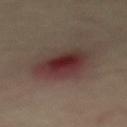The lesion was photographed on a routine skin check and not biopsied; there is no pathology result.
About 6 mm across.
The lesion is on the mid back.
Captured under cross-polarized illumination.
An algorithmic analysis of the crop reported a lesion area of about 19 mm², an eccentricity of roughly 0.75, and a symmetry-axis asymmetry near 0.2. The analysis additionally found an average lesion color of about L≈33 a*≈20 b*≈19 (CIELAB), a lesion–skin lightness drop of about 10, and a normalized border contrast of about 9.5. The analysis additionally found a classifier nevus-likeness of about 0/100 and a detector confidence of about 100 out of 100 that the crop contains a lesion.
A male subject, in their mid-60s.
Cropped from a whole-body photographic skin survey; the tile spans about 15 mm.